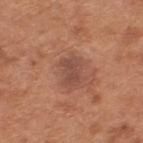Findings:
• notes: catalogued during a skin exam; not biopsied
• anatomic site: the upper back
• subject: male, approximately 65 years of age
• imaging modality: total-body-photography crop, ~15 mm field of view
• lighting: white-light
• image-analysis metrics: a lesion area of about 6.5 mm², an eccentricity of roughly 0.75, and a symmetry-axis asymmetry near 0.25; a nevus-likeness score of about 0/100 and a detector confidence of about 100 out of 100 that the crop contains a lesion